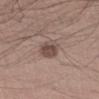Part of a total-body skin-imaging series; this lesion was reviewed on a skin check and was not flagged for biopsy. Cropped from a total-body skin-imaging series; the visible field is about 15 mm. Measured at roughly 3.5 mm in maximum diameter. From the left forearm. The tile uses white-light illumination. A male subject, aged 53 to 57.